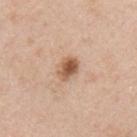acquisition: ~15 mm crop, total-body skin-cancer survey | anatomic site: the chest | TBP lesion metrics: a border-irregularity rating of about 2/10, a color-variation rating of about 5.5/10, and radial color variation of about 2 | subject: male, aged approximately 45.